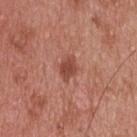Clinical impression:
Part of a total-body skin-imaging series; this lesion was reviewed on a skin check and was not flagged for biopsy.
Background:
A male subject, aged around 55. About 3 mm across. An algorithmic analysis of the crop reported an area of roughly 4.5 mm², an outline eccentricity of about 0.75 (0 = round, 1 = elongated), and a shape-asymmetry score of about 0.2 (0 = symmetric). The analysis additionally found a lesion–skin lightness drop of about 11 and a normalized lesion–skin contrast near 8. The analysis additionally found border irregularity of about 2 on a 0–10 scale, a within-lesion color-variation index near 2/10, and radial color variation of about 0.5. And it measured a detector confidence of about 100 out of 100 that the crop contains a lesion. The tile uses white-light illumination. A 15 mm crop from a total-body photograph taken for skin-cancer surveillance. Located on the upper back.